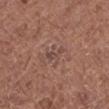<lesion>
  <biopsy_status>not biopsied; imaged during a skin examination</biopsy_status>
  <lighting>white-light</lighting>
  <automated_metrics>
    <cielab_L>45</cielab_L>
    <cielab_a>19</cielab_a>
    <cielab_b>22</cielab_b>
    <vs_skin_darker_L>7.0</vs_skin_darker_L>
    <vs_skin_contrast_norm>5.5</vs_skin_contrast_norm>
    <nevus_likeness_0_100>0</nevus_likeness_0_100>
    <lesion_detection_confidence_0_100>95</lesion_detection_confidence_0_100>
  </automated_metrics>
  <patient>
    <sex>female</sex>
    <age_approx>50</age_approx>
  </patient>
  <site>right lower leg</site>
  <image>
    <source>total-body photography crop</source>
    <field_of_view_mm>15</field_of_view_mm>
  </image>
</lesion>